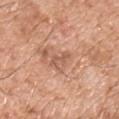The lesion was photographed on a routine skin check and not biopsied; there is no pathology result. The subject is a male aged 73 to 77. On the chest. A 15 mm crop from a total-body photograph taken for skin-cancer surveillance.